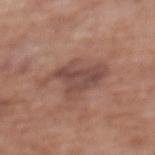workup — total-body-photography surveillance lesion; no biopsy | image — total-body-photography crop, ~15 mm field of view | patient — female, in their mid-70s | lesion size — ~6 mm (longest diameter) | anatomic site — the upper back | tile lighting — white-light | image-analysis metrics — an average lesion color of about L≈47 a*≈20 b*≈24 (CIELAB), about 9 CIELAB-L* units darker than the surrounding skin, and a lesion-to-skin contrast of about 7 (normalized; higher = more distinct); a border-irregularity rating of about 5.5/10, internal color variation of about 4 on a 0–10 scale, and peripheral color asymmetry of about 1.5.